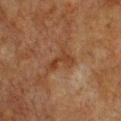Impression: Captured during whole-body skin photography for melanoma surveillance; the lesion was not biopsied. Background: The lesion is on the chest. A 15 mm close-up tile from a total-body photography series done for melanoma screening. A female subject aged 53–57. This is a cross-polarized tile. Approximately 4 mm at its widest.